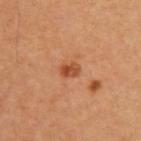Clinical impression:
The lesion was photographed on a routine skin check and not biopsied; there is no pathology result.
Background:
A close-up tile cropped from a whole-body skin photograph, about 15 mm across. Automated image analysis of the tile measured an eccentricity of roughly 0.7 and two-axis asymmetry of about 0.2. The analysis additionally found roughly 10 lightness units darker than nearby skin and a normalized lesion–skin contrast near 8. The lesion's longest dimension is about 2.5 mm. Captured under cross-polarized illumination. From the upper back. The subject is a male about 65 years old.